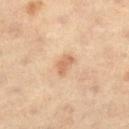A roughly 15 mm field-of-view crop from a total-body skin photograph.
A male patient aged 58 to 62.
On the left thigh.
The recorded lesion diameter is about 2.5 mm.
The lesion-visualizer software estimated a lesion area of about 3 mm², an outline eccentricity of about 0.85 (0 = round, 1 = elongated), and a symmetry-axis asymmetry near 0.3. The software also gave an average lesion color of about L≈60 a*≈20 b*≈33 (CIELAB), about 9 CIELAB-L* units darker than the surrounding skin, and a lesion-to-skin contrast of about 6 (normalized; higher = more distinct).